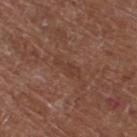Imaged during a routine full-body skin examination; the lesion was not biopsied and no histopathology is available. A male patient aged 73–77. An algorithmic analysis of the crop reported a lesion area of about 3.5 mm², an eccentricity of roughly 0.9, and a shape-asymmetry score of about 0.4 (0 = symmetric). The software also gave border irregularity of about 5 on a 0–10 scale and radial color variation of about 0.5. The software also gave a classifier nevus-likeness of about 0/100 and a lesion-detection confidence of about 100/100. A lesion tile, about 15 mm wide, cut from a 3D total-body photograph. The lesion is located on the left lower leg. Longest diameter approximately 3.5 mm.